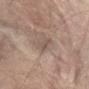<tbp_lesion>
  <biopsy_status>not biopsied; imaged during a skin examination</biopsy_status>
  <lesion_size>
    <long_diameter_mm_approx>2.5</long_diameter_mm_approx>
  </lesion_size>
  <patient>
    <sex>male</sex>
    <age_approx>75</age_approx>
  </patient>
  <lighting>white-light</lighting>
  <site>abdomen</site>
  <image>
    <source>total-body photography crop</source>
    <field_of_view_mm>15</field_of_view_mm>
  </image>
</tbp_lesion>